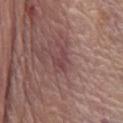biopsy_status: not biopsied; imaged during a skin examination
lesion_size:
  long_diameter_mm_approx: 3.0
lighting: white-light
patient:
  sex: male
  age_approx: 80
image:
  source: total-body photography crop
  field_of_view_mm: 15
automated_metrics:
  cielab_L: 43
  cielab_a: 22
  cielab_b: 18
  vs_skin_darker_L: 7.0
  vs_skin_contrast_norm: 6.0
  nevus_likeness_0_100: 0
  lesion_detection_confidence_0_100: 50
site: chest